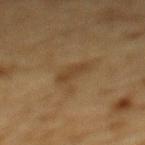Q: Was a biopsy performed?
A: imaged on a skin check; not biopsied
Q: Automated lesion metrics?
A: an eccentricity of roughly 0.9 and two-axis asymmetry of about 0.3; a mean CIELAB color near L≈35 a*≈13 b*≈29 and a lesion–skin lightness drop of about 6; border irregularity of about 3.5 on a 0–10 scale, a color-variation rating of about 1.5/10, and peripheral color asymmetry of about 0; a classifier nevus-likeness of about 5/100 and a detector confidence of about 95 out of 100 that the crop contains a lesion
Q: Where on the body is the lesion?
A: the mid back
Q: Who is the patient?
A: male, aged around 85 or older
Q: How was this image acquired?
A: total-body-photography crop, ~15 mm field of view